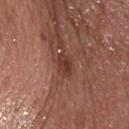Captured during whole-body skin photography for melanoma surveillance; the lesion was not biopsied. The patient is a male approximately 55 years of age. The recorded lesion diameter is about 3 mm. Captured under white-light illumination. Cropped from a total-body skin-imaging series; the visible field is about 15 mm. The lesion is located on the chest.